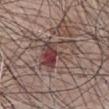Located on the chest.
Cropped from a whole-body photographic skin survey; the tile spans about 15 mm.
The subject is a male aged 68–72.
Approximately 5.5 mm at its widest.